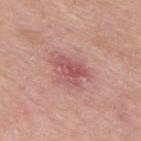Background: The total-body-photography lesion software estimated a lesion area of about 9 mm², a shape eccentricity near 0.7, and two-axis asymmetry of about 0.45. The software also gave a lesion color around L≈55 a*≈28 b*≈23 in CIELAB and a lesion–skin lightness drop of about 9. The analysis additionally found a border-irregularity index near 5/10, a within-lesion color-variation index near 4/10, and a peripheral color-asymmetry measure near 1.5. The software also gave an automated nevus-likeness rating near 0 out of 100. Cropped from a total-body skin-imaging series; the visible field is about 15 mm. A male subject, approximately 55 years of age. On the mid back. The recorded lesion diameter is about 4.5 mm.